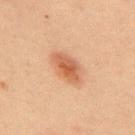<tbp_lesion>
  <biopsy_status>not biopsied; imaged during a skin examination</biopsy_status>
  <patient>
    <sex>male</sex>
    <age_approx>60</age_approx>
  </patient>
  <site>mid back</site>
  <image>
    <source>total-body photography crop</source>
    <field_of_view_mm>15</field_of_view_mm>
  </image>
</tbp_lesion>